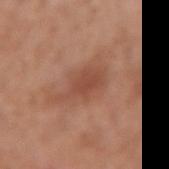notes: catalogued during a skin exam; not biopsied
location: the arm
size: ≈5.5 mm
subject: female, about 50 years old
lighting: white-light illumination
image: total-body-photography crop, ~15 mm field of view
image-analysis metrics: a lesion area of about 10 mm², a shape eccentricity near 0.85, and a shape-asymmetry score of about 0.35 (0 = symmetric); a border-irregularity index near 4.5/10 and internal color variation of about 2 on a 0–10 scale; a detector confidence of about 100 out of 100 that the crop contains a lesion